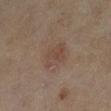Case summary:
- biopsy status: no biopsy performed (imaged during a skin exam)
- site: the left lower leg
- imaging modality: ~15 mm crop, total-body skin-cancer survey
- diameter: ≈3 mm
- subject: female, aged around 60